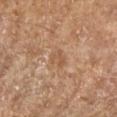Assessment:
Imaged during a routine full-body skin examination; the lesion was not biopsied and no histopathology is available.
Clinical summary:
Cropped from a total-body skin-imaging series; the visible field is about 15 mm. Located on the right forearm. A female subject in their 70s. Measured at roughly 2.5 mm in maximum diameter.